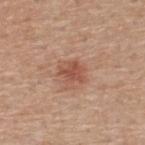Part of a total-body skin-imaging series; this lesion was reviewed on a skin check and was not flagged for biopsy. Cropped from a whole-body photographic skin survey; the tile spans about 15 mm. The lesion is on the back. Approximately 3 mm at its widest. A male subject, aged approximately 60. This is a white-light tile.